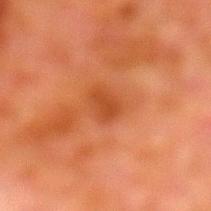Captured during whole-body skin photography for melanoma surveillance; the lesion was not biopsied. Located on the leg. The patient is a male in their 80s. Captured under cross-polarized illumination. The lesion-visualizer software estimated an area of roughly 3.5 mm², an outline eccentricity of about 0.8 (0 = round, 1 = elongated), and a shape-asymmetry score of about 0.3 (0 = symmetric). And it measured a nevus-likeness score of about 0/100 and a detector confidence of about 100 out of 100 that the crop contains a lesion. Measured at roughly 2.5 mm in maximum diameter. A 15 mm close-up extracted from a 3D total-body photography capture.